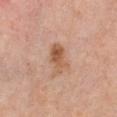{"biopsy_status": "not biopsied; imaged during a skin examination"}